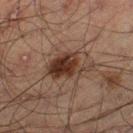workup: imaged on a skin check; not biopsied
patient: male, aged approximately 50
anatomic site: the left thigh
imaging modality: 15 mm crop, total-body photography
lighting: cross-polarized
automated metrics: a footprint of about 11 mm², an eccentricity of roughly 0.75, and two-axis asymmetry of about 0.4; a lesion color around L≈26 a*≈15 b*≈21 in CIELAB, roughly 11 lightness units darker than nearby skin, and a lesion-to-skin contrast of about 11 (normalized; higher = more distinct)